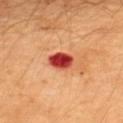Impression:
The lesion was photographed on a routine skin check and not biopsied; there is no pathology result.
Image and clinical context:
The lesion's longest dimension is about 3 mm. The total-body-photography lesion software estimated a mean CIELAB color near L≈45 a*≈42 b*≈36, about 21 CIELAB-L* units darker than the surrounding skin, and a normalized lesion–skin contrast near 14. The software also gave a nevus-likeness score of about 0/100 and lesion-presence confidence of about 100/100. Cropped from a whole-body photographic skin survey; the tile spans about 15 mm. The lesion is on the upper back. The tile uses cross-polarized illumination. A male subject, aged around 30.